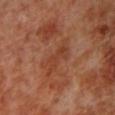Imaged during a routine full-body skin examination; the lesion was not biopsied and no histopathology is available. A male patient, approximately 70 years of age. Automated image analysis of the tile measured a lesion area of about 5 mm² and a symmetry-axis asymmetry near 0.4. It also reported a border-irregularity index near 5/10 and a within-lesion color-variation index near 1.5/10. This image is a 15 mm lesion crop taken from a total-body photograph. Longest diameter approximately 4 mm. Located on the left lower leg.